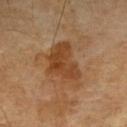{
  "biopsy_status": "not biopsied; imaged during a skin examination",
  "image": {
    "source": "total-body photography crop",
    "field_of_view_mm": 15
  },
  "automated_metrics": {
    "border_irregularity_0_10": 4.0,
    "color_variation_0_10": 4.0,
    "peripheral_color_asymmetry": 1.5,
    "nevus_likeness_0_100": 0,
    "lesion_detection_confidence_0_100": 100
  },
  "patient": {
    "sex": "male",
    "age_approx": 70
  },
  "lesion_size": {
    "long_diameter_mm_approx": 6.0
  },
  "site": "right upper arm"
}